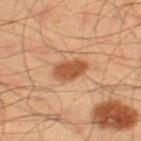A male patient, aged 43 to 47. The lesion is located on the left thigh. The tile uses cross-polarized illumination. A 15 mm close-up extracted from a 3D total-body photography capture.